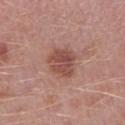- notes — total-body-photography surveillance lesion; no biopsy
- lighting — white-light
- acquisition — ~15 mm tile from a whole-body skin photo
- patient — male, aged around 50
- location — the right lower leg